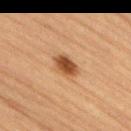Clinical impression: The lesion was tiled from a total-body skin photograph and was not biopsied. Acquisition and patient details: Captured under cross-polarized illumination. The patient is a male aged 83 or older. The lesion is located on the lower back. A roughly 15 mm field-of-view crop from a total-body skin photograph.